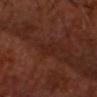Assessment: Imaged during a routine full-body skin examination; the lesion was not biopsied and no histopathology is available. Acquisition and patient details: Captured under cross-polarized illumination. Cropped from a whole-body photographic skin survey; the tile spans about 15 mm. The patient is a male aged approximately 60. Located on the head or neck. An algorithmic analysis of the crop reported a mean CIELAB color near L≈22 a*≈22 b*≈23 and a normalized lesion–skin contrast near 5. The analysis additionally found lesion-presence confidence of about 70/100.